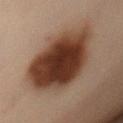Imaged during a routine full-body skin examination; the lesion was not biopsied and no histopathology is available. A female patient, aged around 45. On the front of the torso. Cropped from a total-body skin-imaging series; the visible field is about 15 mm.